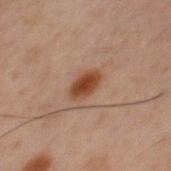workup: total-body-photography surveillance lesion; no biopsy
tile lighting: cross-polarized illumination
acquisition: ~15 mm crop, total-body skin-cancer survey
patient: male, in their 60s
automated lesion analysis: a mean CIELAB color near L≈33 a*≈18 b*≈25, a lesion–skin lightness drop of about 10, and a normalized border contrast of about 10.5
body site: the chest
lesion diameter: ≈3 mm